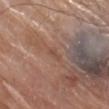Recorded during total-body skin imaging; not selected for excision or biopsy.
Captured under white-light illumination.
A male subject aged 78 to 82.
Measured at roughly 2 mm in maximum diameter.
Cropped from a total-body skin-imaging series; the visible field is about 15 mm.
The lesion is on the arm.